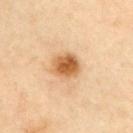Findings:
* follow-up: total-body-photography surveillance lesion; no biopsy
* lighting: cross-polarized illumination
* patient: male, aged around 55
* site: the chest
* automated lesion analysis: an eccentricity of roughly 0.55 and a symmetry-axis asymmetry near 0.15; border irregularity of about 1.5 on a 0–10 scale, a color-variation rating of about 4.5/10, and radial color variation of about 1.5; lesion-presence confidence of about 100/100
* lesion size: ~3.5 mm (longest diameter)
* imaging modality: ~15 mm crop, total-body skin-cancer survey